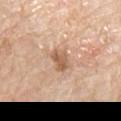Q: Was a biopsy performed?
A: imaged on a skin check; not biopsied
Q: Patient demographics?
A: male, about 60 years old
Q: What is the anatomic site?
A: the chest
Q: What is the imaging modality?
A: 15 mm crop, total-body photography
Q: Automated lesion metrics?
A: a lesion area of about 5 mm², a shape eccentricity near 0.7, and a shape-asymmetry score of about 0.25 (0 = symmetric)
Q: What lighting was used for the tile?
A: white-light
Q: How large is the lesion?
A: about 3 mm